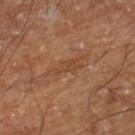{"biopsy_status": "not biopsied; imaged during a skin examination", "image": {"source": "total-body photography crop", "field_of_view_mm": 15}, "site": "right lower leg", "patient": {"sex": "male", "age_approx": 60}, "automated_metrics": {"area_mm2_approx": 4.5, "eccentricity": 0.9, "border_irregularity_0_10": 6.5, "color_variation_0_10": 1.5}}